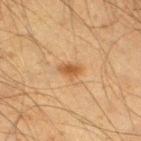The lesion was photographed on a routine skin check and not biopsied; there is no pathology result.
The tile uses cross-polarized illumination.
A male patient, aged around 60.
A 15 mm close-up tile from a total-body photography series done for melanoma screening.
Located on the right thigh.
Longest diameter approximately 2.5 mm.